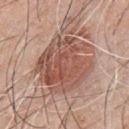Q: Was this lesion biopsied?
A: imaged on a skin check; not biopsied
Q: What lighting was used for the tile?
A: white-light illumination
Q: What is the imaging modality?
A: total-body-photography crop, ~15 mm field of view
Q: Where on the body is the lesion?
A: the chest
Q: What is the lesion's diameter?
A: about 7 mm
Q: Who is the patient?
A: male, aged approximately 45